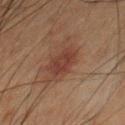Case summary:
• workup: catalogued during a skin exam; not biopsied
• acquisition: ~15 mm crop, total-body skin-cancer survey
• subject: male, roughly 50 years of age
• lighting: cross-polarized
• body site: the chest